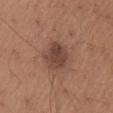Notes:
– body site · the front of the torso
– illumination · white-light
– TBP lesion metrics · a footprint of about 9 mm², a shape eccentricity near 0.7, and a symmetry-axis asymmetry near 0.2; an average lesion color of about L≈43 a*≈20 b*≈25 (CIELAB); a nevus-likeness score of about 50/100 and a lesion-detection confidence of about 100/100
– image source · 15 mm crop, total-body photography
– size · about 4 mm
– patient · female, about 65 years old